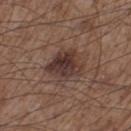Notes:
• follow-up · total-body-photography surveillance lesion; no biopsy
• image source · total-body-photography crop, ~15 mm field of view
• TBP lesion metrics · a footprint of about 11 mm², a shape eccentricity near 0.6, and two-axis asymmetry of about 0.35; a lesion color around L≈35 a*≈17 b*≈21 in CIELAB, a lesion–skin lightness drop of about 11, and a normalized lesion–skin contrast near 10; an automated nevus-likeness rating near 75 out of 100 and a lesion-detection confidence of about 100/100
• anatomic site · the leg
• subject · male, in their mid- to late 50s
• lighting · white-light
• diameter · ~4.5 mm (longest diameter)